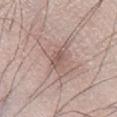Context: Cropped from a total-body skin-imaging series; the visible field is about 15 mm. Automated image analysis of the tile measured a nevus-likeness score of about 0/100. On the right lower leg. The tile uses white-light illumination. A male patient, aged around 25. About 2.5 mm across.